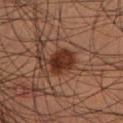Background: About 4 mm across. Located on the leg. A male patient approximately 50 years of age. This is a cross-polarized tile. The lesion-visualizer software estimated a footprint of about 8.5 mm², a shape eccentricity near 0.6, and two-axis asymmetry of about 0.2. This image is a 15 mm lesion crop taken from a total-body photograph.